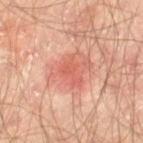follow-up = total-body-photography surveillance lesion; no biopsy
image source = ~15 mm tile from a whole-body skin photo
patient = male, approximately 65 years of age
site = the left thigh